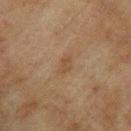Captured during whole-body skin photography for melanoma surveillance; the lesion was not biopsied. Captured under cross-polarized illumination. Measured at roughly 2.5 mm in maximum diameter. A lesion tile, about 15 mm wide, cut from a 3D total-body photograph. On the arm. The total-body-photography lesion software estimated a shape-asymmetry score of about 0.2 (0 = symmetric). The software also gave a lesion color around L≈40 a*≈14 b*≈28 in CIELAB, roughly 5 lightness units darker than nearby skin, and a normalized border contrast of about 5.5. The software also gave a classifier nevus-likeness of about 0/100 and a lesion-detection confidence of about 100/100. A male patient, aged 73–77.